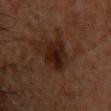The lesion was photographed on a routine skin check and not biopsied; there is no pathology result. The lesion's longest dimension is about 3.5 mm. The subject is a male in their 50s. The lesion is on the front of the torso. Automated tile analysis of the lesion measured a lesion area of about 8.5 mm² and an eccentricity of roughly 0.55. It also reported about 7 CIELAB-L* units darker than the surrounding skin and a lesion-to-skin contrast of about 10.5 (normalized; higher = more distinct). The software also gave border irregularity of about 3 on a 0–10 scale, a within-lesion color-variation index near 3.5/10, and peripheral color asymmetry of about 1. A 15 mm close-up tile from a total-body photography series done for melanoma screening. Captured under cross-polarized illumination.